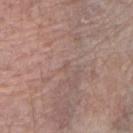This lesion was catalogued during total-body skin photography and was not selected for biopsy.
Imaged with white-light lighting.
A 15 mm close-up extracted from a 3D total-body photography capture.
The lesion is on the arm.
Approximately 1 mm at its widest.
The patient is a female aged 63 to 67.
An algorithmic analysis of the crop reported a shape eccentricity near 0.75. And it measured a nevus-likeness score of about 0/100 and a detector confidence of about 50 out of 100 that the crop contains a lesion.